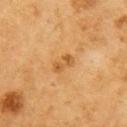<record>
  <biopsy_status>not biopsied; imaged during a skin examination</biopsy_status>
  <lesion_size>
    <long_diameter_mm_approx>2.5</long_diameter_mm_approx>
  </lesion_size>
  <patient>
    <sex>male</sex>
    <age_approx>60</age_approx>
  </patient>
  <site>arm</site>
  <image>
    <source>total-body photography crop</source>
    <field_of_view_mm>15</field_of_view_mm>
  </image>
  <lighting>cross-polarized</lighting>
</record>